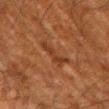Acquisition and patient details: Captured under cross-polarized illumination. The patient is a male aged approximately 60. The lesion's longest dimension is about 3.5 mm. Cropped from a total-body skin-imaging series; the visible field is about 15 mm. The lesion is located on the left upper arm.